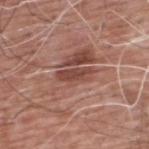Clinical summary: A male subject aged 58–62. The total-body-photography lesion software estimated an eccentricity of roughly 0.9 and two-axis asymmetry of about 0.25. The software also gave a mean CIELAB color near L≈45 a*≈24 b*≈25, roughly 11 lightness units darker than nearby skin, and a lesion-to-skin contrast of about 8 (normalized; higher = more distinct). This image is a 15 mm lesion crop taken from a total-body photograph. On the upper back. Captured under white-light illumination.